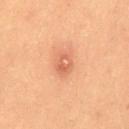Imaged during a routine full-body skin examination; the lesion was not biopsied and no histopathology is available.
Imaged with cross-polarized lighting.
The subject is a female in their 20s.
A 15 mm close-up extracted from a 3D total-body photography capture.
Automated tile analysis of the lesion measured an eccentricity of roughly 0.7 and two-axis asymmetry of about 0.15. The analysis additionally found a border-irregularity index near 1.5/10, a within-lesion color-variation index near 5/10, and peripheral color asymmetry of about 2. The software also gave a classifier nevus-likeness of about 5/100.
On the back.